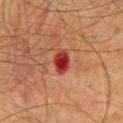biopsy_status: not biopsied; imaged during a skin examination
site: mid back
lighting: cross-polarized
patient:
  sex: male
  age_approx: 65
image:
  source: total-body photography crop
  field_of_view_mm: 15
lesion_size:
  long_diameter_mm_approx: 3.0